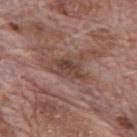<tbp_lesion>
  <biopsy_status>not biopsied; imaged during a skin examination</biopsy_status>
  <lighting>white-light</lighting>
  <site>mid back</site>
  <automated_metrics>
    <area_mm2_approx>8.0</area_mm2_approx>
    <eccentricity>0.75</eccentricity>
    <shape_asymmetry>0.4</shape_asymmetry>
    <border_irregularity_0_10>4.5</border_irregularity_0_10>
    <color_variation_0_10>5.5</color_variation_0_10>
    <nevus_likeness_0_100>0</nevus_likeness_0_100>
    <lesion_detection_confidence_0_100>85</lesion_detection_confidence_0_100>
  </automated_metrics>
  <image>
    <source>total-body photography crop</source>
    <field_of_view_mm>15</field_of_view_mm>
  </image>
  <patient>
    <sex>male</sex>
    <age_approx>70</age_approx>
  </patient>
  <lesion_size>
    <long_diameter_mm_approx>4.5</long_diameter_mm_approx>
  </lesion_size>
</tbp_lesion>